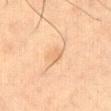| field | value |
|---|---|
| workup | imaged on a skin check; not biopsied |
| body site | the front of the torso |
| subject | male, roughly 55 years of age |
| image source | ~15 mm tile from a whole-body skin photo |
| diameter | ~2.5 mm (longest diameter) |
| automated lesion analysis | border irregularity of about 2 on a 0–10 scale and a peripheral color-asymmetry measure near 0.5; an automated nevus-likeness rating near 0 out of 100 and lesion-presence confidence of about 70/100 |